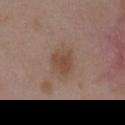No biopsy was performed on this lesion — it was imaged during a full skin examination and was not determined to be concerning.
On the chest.
A female subject about 35 years old.
Cropped from a whole-body photographic skin survey; the tile spans about 15 mm.